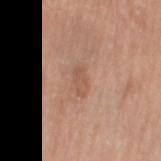follow-up: catalogued during a skin exam; not biopsied
image: ~15 mm tile from a whole-body skin photo
lighting: white-light illumination
body site: the left thigh
automated metrics: an outline eccentricity of about 0.9 (0 = round, 1 = elongated) and a symmetry-axis asymmetry near 0.3; a within-lesion color-variation index near 0.5/10; a nevus-likeness score of about 0/100
subject: female, aged around 55